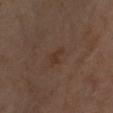{"biopsy_status": "not biopsied; imaged during a skin examination", "site": "left forearm", "patient": {"sex": "female", "age_approx": 55}, "lesion_size": {"long_diameter_mm_approx": 2.5}, "image": {"source": "total-body photography crop", "field_of_view_mm": 15}, "lighting": "cross-polarized"}